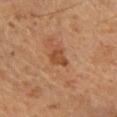{
  "lighting": "cross-polarized",
  "image": {
    "source": "total-body photography crop",
    "field_of_view_mm": 15
  },
  "patient": {
    "sex": "male",
    "age_approx": 65
  },
  "lesion_size": {
    "long_diameter_mm_approx": 2.5
  },
  "site": "right lower leg",
  "automated_metrics": {
    "area_mm2_approx": 4.0,
    "eccentricity": 0.65,
    "shape_asymmetry": 0.4,
    "cielab_L": 41,
    "cielab_a": 22,
    "cielab_b": 31,
    "vs_skin_darker_L": 8.0,
    "vs_skin_contrast_norm": 7.0,
    "border_irregularity_0_10": 4.0,
    "peripheral_color_asymmetry": 0.5
  }
}